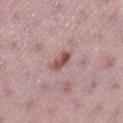The lesion was photographed on a routine skin check and not biopsied; there is no pathology result. A region of skin cropped from a whole-body photographic capture, roughly 15 mm wide. Captured under white-light illumination. Automated image analysis of the tile measured a mean CIELAB color near L≈52 a*≈22 b*≈21, about 12 CIELAB-L* units darker than the surrounding skin, and a normalized lesion–skin contrast near 8.5. The software also gave a border-irregularity index near 2.5/10, internal color variation of about 4.5 on a 0–10 scale, and a peripheral color-asymmetry measure near 2. It also reported a nevus-likeness score of about 10/100 and lesion-presence confidence of about 100/100. The recorded lesion diameter is about 3 mm. A female patient aged approximately 40. The lesion is located on the leg.